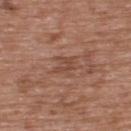Notes:
- acquisition: ~15 mm crop, total-body skin-cancer survey
- patient: male, aged around 50
- body site: the upper back
- automated metrics: a lesion area of about 3 mm², an outline eccentricity of about 0.75 (0 = round, 1 = elongated), and two-axis asymmetry of about 0.5; an automated nevus-likeness rating near 0 out of 100 and a lesion-detection confidence of about 100/100
- tile lighting: white-light illumination